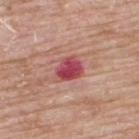notes: no biopsy performed (imaged during a skin exam) | subject: male, aged 63–67 | image: ~15 mm tile from a whole-body skin photo | site: the upper back.